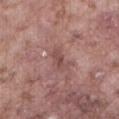Case summary:
• acquisition: ~15 mm tile from a whole-body skin photo
• lesion size: about 3 mm
• body site: the front of the torso
• patient: male, in their mid-70s
• illumination: white-light illumination
• TBP lesion metrics: an area of roughly 4 mm², a shape eccentricity near 0.85, and two-axis asymmetry of about 0.25; an average lesion color of about L≈48 a*≈21 b*≈22 (CIELAB) and a normalized lesion–skin contrast near 6; an automated nevus-likeness rating near 0 out of 100 and a lesion-detection confidence of about 90/100